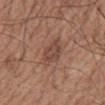The lesion was tiled from a total-body skin photograph and was not biopsied.